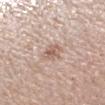Findings:
* biopsy status · no biopsy performed (imaged during a skin exam)
* image · 15 mm crop, total-body photography
* body site · the arm
* subject · female, aged 48–52
* diameter · ≈3 mm